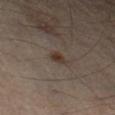Assessment:
Captured during whole-body skin photography for melanoma surveillance; the lesion was not biopsied.
Context:
A male subject, aged 53 to 57. The lesion is on the left lower leg. The lesion's longest dimension is about 2 mm. A region of skin cropped from a whole-body photographic capture, roughly 15 mm wide.